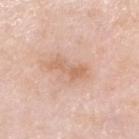* workup — imaged on a skin check; not biopsied
* body site — the head or neck
* lesion size — about 4.5 mm
* patient — male, aged 78–82
* imaging modality — 15 mm crop, total-body photography
* TBP lesion metrics — a footprint of about 6 mm²; a mean CIELAB color near L≈65 a*≈20 b*≈32 and a normalized lesion–skin contrast near 6; border irregularity of about 5 on a 0–10 scale, a within-lesion color-variation index near 1.5/10, and a peripheral color-asymmetry measure near 0.5; an automated nevus-likeness rating near 0 out of 100
* lighting — white-light illumination